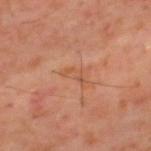| field | value |
|---|---|
| follow-up | total-body-photography surveillance lesion; no biopsy |
| lighting | cross-polarized |
| acquisition | 15 mm crop, total-body photography |
| image-analysis metrics | a lesion area of about 3 mm², an outline eccentricity of about 0.85 (0 = round, 1 = elongated), and a shape-asymmetry score of about 0.5 (0 = symmetric); a mean CIELAB color near L≈50 a*≈23 b*≈33, roughly 5 lightness units darker than nearby skin, and a normalized lesion–skin contrast near 4.5; border irregularity of about 5.5 on a 0–10 scale and radial color variation of about 0; a classifier nevus-likeness of about 0/100 and a lesion-detection confidence of about 100/100 |
| anatomic site | the upper back |
| subject | male, roughly 60 years of age |